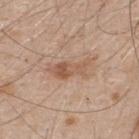Q: Is there a histopathology result?
A: imaged on a skin check; not biopsied
Q: Who is the patient?
A: male, about 50 years old
Q: Automated lesion metrics?
A: a lesion color around L≈56 a*≈19 b*≈31 in CIELAB and a lesion-to-skin contrast of about 7 (normalized; higher = more distinct); internal color variation of about 5 on a 0–10 scale and peripheral color asymmetry of about 2
Q: What is the anatomic site?
A: the upper back
Q: What is the lesion's diameter?
A: ~5 mm (longest diameter)
Q: How was this image acquired?
A: ~15 mm crop, total-body skin-cancer survey
Q: Illumination type?
A: white-light